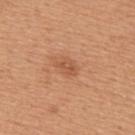Impression:
This lesion was catalogued during total-body skin photography and was not selected for biopsy.
Clinical summary:
From the upper back. This image is a 15 mm lesion crop taken from a total-body photograph. A male patient, aged 53–57.